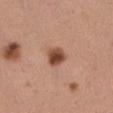Clinical impression:
The lesion was tiled from a total-body skin photograph and was not biopsied.
Acquisition and patient details:
A region of skin cropped from a whole-body photographic capture, roughly 15 mm wide. A female subject aged 28 to 32. Measured at roughly 3 mm in maximum diameter. From the left thigh.